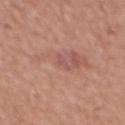Context: Located on the chest. The subject is a male in their mid- to late 40s. Imaged with white-light lighting. Longest diameter approximately 3 mm. Cropped from a whole-body photographic skin survey; the tile spans about 15 mm.